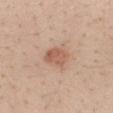biopsy status: total-body-photography surveillance lesion; no biopsy | subject: female, aged around 40 | imaging modality: 15 mm crop, total-body photography | size: about 3 mm | body site: the mid back | illumination: white-light illumination.